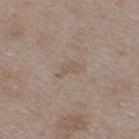Recorded during total-body skin imaging; not selected for excision or biopsy.
Measured at roughly 2.5 mm in maximum diameter.
A roughly 15 mm field-of-view crop from a total-body skin photograph.
The total-body-photography lesion software estimated a footprint of about 2.5 mm², an outline eccentricity of about 0.9 (0 = round, 1 = elongated), and a shape-asymmetry score of about 0.5 (0 = symmetric). The analysis additionally found an average lesion color of about L≈54 a*≈13 b*≈24 (CIELAB). The analysis additionally found a nevus-likeness score of about 0/100.
A male patient about 50 years old.
Captured under white-light illumination.
The lesion is on the right thigh.